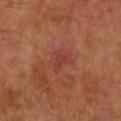Assessment:
The lesion was photographed on a routine skin check and not biopsied; there is no pathology result.
Context:
The patient is a male about 55 years old. Longest diameter approximately 3 mm. Cropped from a total-body skin-imaging series; the visible field is about 15 mm. An algorithmic analysis of the crop reported a lesion color around L≈40 a*≈29 b*≈27 in CIELAB, roughly 6 lightness units darker than nearby skin, and a normalized lesion–skin contrast near 5. The analysis additionally found a border-irregularity rating of about 3.5/10 and a within-lesion color-variation index near 2/10. This is a cross-polarized tile. From the right forearm.